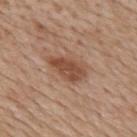biopsy status: total-body-photography surveillance lesion; no biopsy | illumination: white-light illumination | size: about 4.5 mm | site: the upper back | patient: female, aged 48–52 | image: total-body-photography crop, ~15 mm field of view.